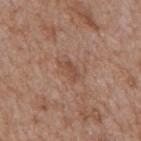Notes:
• follow-up — imaged on a skin check; not biopsied
• imaging modality — 15 mm crop, total-body photography
• lighting — white-light
• location — the mid back
• patient — male, aged approximately 65